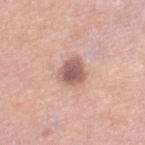Impression:
Captured during whole-body skin photography for melanoma surveillance; the lesion was not biopsied.
Context:
The lesion is located on the right lower leg. A region of skin cropped from a whole-body photographic capture, roughly 15 mm wide. The lesion-visualizer software estimated border irregularity of about 2 on a 0–10 scale and a within-lesion color-variation index near 3.5/10. The software also gave an automated nevus-likeness rating near 45 out of 100 and a detector confidence of about 100 out of 100 that the crop contains a lesion. A male subject, in their 50s.